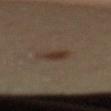Captured during whole-body skin photography for melanoma surveillance; the lesion was not biopsied. A female patient in their mid-60s. Automated tile analysis of the lesion measured border irregularity of about 2 on a 0–10 scale, internal color variation of about 2.5 on a 0–10 scale, and a peripheral color-asymmetry measure near 1. The analysis additionally found a detector confidence of about 100 out of 100 that the crop contains a lesion. A roughly 15 mm field-of-view crop from a total-body skin photograph. From the back. Approximately 3 mm at its widest.